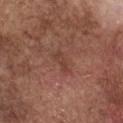{
  "biopsy_status": "not biopsied; imaged during a skin examination",
  "lighting": "white-light",
  "patient": {
    "sex": "male",
    "age_approx": 75
  },
  "image": {
    "source": "total-body photography crop",
    "field_of_view_mm": 15
  },
  "lesion_size": {
    "long_diameter_mm_approx": 2.5
  },
  "site": "chest"
}